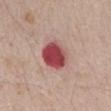Part of a total-body skin-imaging series; this lesion was reviewed on a skin check and was not flagged for biopsy. This is a white-light tile. A 15 mm close-up tile from a total-body photography series done for melanoma screening. The subject is a male in their mid-60s. Measured at roughly 4 mm in maximum diameter. Located on the chest.